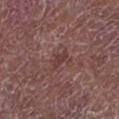Notes:
* patient · male, aged approximately 65
* tile lighting · white-light
* lesion diameter · ~2.5 mm (longest diameter)
* body site · the left lower leg
* acquisition · 15 mm crop, total-body photography